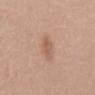  biopsy_status: not biopsied; imaged during a skin examination
  site: mid back
  automated_metrics:
    vs_skin_darker_L: 8.0
    vs_skin_contrast_norm: 6.0
    border_irregularity_0_10: 2.5
    peripheral_color_asymmetry: 0.5
    nevus_likeness_0_100: 25
    lesion_detection_confidence_0_100: 100
  lighting: white-light
  lesion_size:
    long_diameter_mm_approx: 3.0
  patient:
    sex: male
    age_approx: 50
  image:
    source: total-body photography crop
    field_of_view_mm: 15